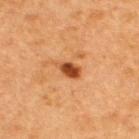This lesion was catalogued during total-body skin photography and was not selected for biopsy. About 3 mm across. An algorithmic analysis of the crop reported a mean CIELAB color near L≈42 a*≈26 b*≈37, a lesion–skin lightness drop of about 14, and a normalized border contrast of about 10.5. The analysis additionally found a nevus-likeness score of about 95/100 and lesion-presence confidence of about 100/100. This is a cross-polarized tile. A female patient in their 40s. A 15 mm crop from a total-body photograph taken for skin-cancer surveillance. The lesion is on the upper back.